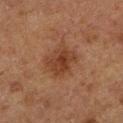Image and clinical context: The lesion is located on the left lower leg. A male patient aged 73 to 77. Imaged with cross-polarized lighting. A 15 mm close-up extracted from a 3D total-body photography capture. The total-body-photography lesion software estimated an automated nevus-likeness rating near 30 out of 100 and lesion-presence confidence of about 100/100.